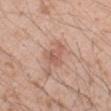Case summary:
• body site · the right forearm
• image · 15 mm crop, total-body photography
• patient · male, aged approximately 25
• lesion size · about 3 mm